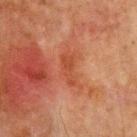follow-up: total-body-photography surveillance lesion; no biopsy
image-analysis metrics: roughly 6 lightness units darker than nearby skin; a classifier nevus-likeness of about 0/100
patient: male, approximately 65 years of age
diameter: ~3.5 mm (longest diameter)
anatomic site: the chest
illumination: cross-polarized illumination
acquisition: total-body-photography crop, ~15 mm field of view